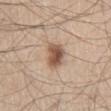Q: Was a biopsy performed?
A: catalogued during a skin exam; not biopsied
Q: How was this image acquired?
A: total-body-photography crop, ~15 mm field of view
Q: Where on the body is the lesion?
A: the right thigh
Q: Patient demographics?
A: male, aged approximately 60